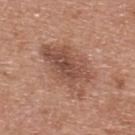Clinical impression:
The lesion was photographed on a routine skin check and not biopsied; there is no pathology result.
Background:
Located on the back. Automated image analysis of the tile measured a footprint of about 21 mm² and an outline eccentricity of about 0.8 (0 = round, 1 = elongated). The analysis additionally found an average lesion color of about L≈50 a*≈22 b*≈28 (CIELAB), about 10 CIELAB-L* units darker than the surrounding skin, and a normalized lesion–skin contrast near 7.5. And it measured an automated nevus-likeness rating near 20 out of 100 and a detector confidence of about 100 out of 100 that the crop contains a lesion. A lesion tile, about 15 mm wide, cut from a 3D total-body photograph. The subject is a male roughly 30 years of age. Captured under white-light illumination. The recorded lesion diameter is about 7 mm.